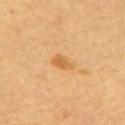  image:
    source: total-body photography crop
    field_of_view_mm: 15
  automated_metrics:
    area_mm2_approx: 3.5
    eccentricity: 0.85
    cielab_L: 55
    cielab_a: 20
    cielab_b: 40
    nevus_likeness_0_100: 50
    lesion_detection_confidence_0_100: 100
  lesion_size:
    long_diameter_mm_approx: 3.0
  patient:
    sex: female
    age_approx: 60
  site: upper back
  lighting: cross-polarized